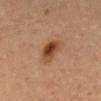| feature | finding |
|---|---|
| workup | imaged on a skin check; not biopsied |
| location | the abdomen |
| patient | female, about 60 years old |
| image source | 15 mm crop, total-body photography |
| illumination | cross-polarized |
| lesion size | about 4 mm |